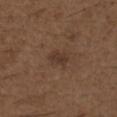No biopsy was performed on this lesion — it was imaged during a full skin examination and was not determined to be concerning. The lesion is located on the chest. Captured under white-light illumination. A male patient, aged 48–52. A lesion tile, about 15 mm wide, cut from a 3D total-body photograph. Automated tile analysis of the lesion measured an outline eccentricity of about 0.85 (0 = round, 1 = elongated) and a shape-asymmetry score of about 0.25 (0 = symmetric). It also reported a within-lesion color-variation index near 1.5/10 and radial color variation of about 0.5. And it measured an automated nevus-likeness rating near 40 out of 100 and a lesion-detection confidence of about 100/100.